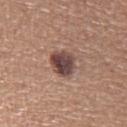The lesion was tiled from a total-body skin photograph and was not biopsied.